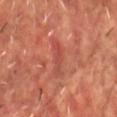Q: Was this lesion biopsied?
A: catalogued during a skin exam; not biopsied
Q: What are the patient's age and sex?
A: male, aged 63 to 67
Q: Lesion size?
A: about 4.5 mm
Q: What is the imaging modality?
A: ~15 mm crop, total-body skin-cancer survey
Q: Lesion location?
A: the upper back
Q: What did automated image analysis measure?
A: a footprint of about 5 mm², an outline eccentricity of about 0.95 (0 = round, 1 = elongated), and a symmetry-axis asymmetry near 0.4
Q: How was the tile lit?
A: cross-polarized